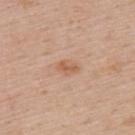The lesion was photographed on a routine skin check and not biopsied; there is no pathology result. This is a white-light tile. On the upper back. The patient is a male in their mid-50s. An algorithmic analysis of the crop reported a color-variation rating of about 2/10 and a peripheral color-asymmetry measure near 1. It also reported a nevus-likeness score of about 5/100 and lesion-presence confidence of about 100/100. A lesion tile, about 15 mm wide, cut from a 3D total-body photograph. The recorded lesion diameter is about 2.5 mm.